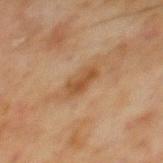Measured at roughly 3.5 mm in maximum diameter. On the mid back. Cropped from a whole-body photographic skin survey; the tile spans about 15 mm. A male patient, roughly 70 years of age.